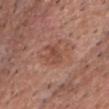The lesion was photographed on a routine skin check and not biopsied; there is no pathology result. Imaged with white-light lighting. About 3 mm across. A 15 mm crop from a total-body photograph taken for skin-cancer surveillance. A male subject aged 78 to 82. The total-body-photography lesion software estimated a footprint of about 4 mm², an outline eccentricity of about 0.75 (0 = round, 1 = elongated), and a symmetry-axis asymmetry near 0.4. And it measured a nevus-likeness score of about 0/100 and lesion-presence confidence of about 100/100. Located on the head or neck.